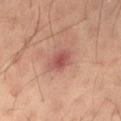Recorded during total-body skin imaging; not selected for excision or biopsy.
A 15 mm crop from a total-body photograph taken for skin-cancer surveillance.
A male subject, in their mid-30s.
The lesion is on the back.
Imaged with cross-polarized lighting.